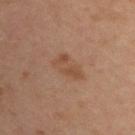– workup · no biopsy performed (imaged during a skin exam)
– body site · the upper back
– size · about 4 mm
– lighting · cross-polarized
– patient · male, aged around 35
– image source · 15 mm crop, total-body photography